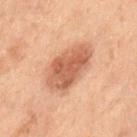This lesion was catalogued during total-body skin photography and was not selected for biopsy. This is a cross-polarized tile. A male subject, about 70 years old. On the left thigh. Cropped from a total-body skin-imaging series; the visible field is about 15 mm.